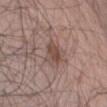workup — total-body-photography surveillance lesion; no biopsy
image — 15 mm crop, total-body photography
patient — male, aged approximately 65
lesion size — about 3 mm
anatomic site — the chest
illumination — white-light illumination
automated lesion analysis — a lesion color around L≈47 a*≈18 b*≈23 in CIELAB and about 9 CIELAB-L* units darker than the surrounding skin; border irregularity of about 3 on a 0–10 scale and radial color variation of about 1; an automated nevus-likeness rating near 45 out of 100 and a detector confidence of about 100 out of 100 that the crop contains a lesion